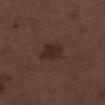Captured during whole-body skin photography for melanoma surveillance; the lesion was not biopsied. Captured under white-light illumination. About 2.5 mm across. The subject is a male aged approximately 70. A 15 mm close-up extracted from a 3D total-body photography capture. The lesion is located on the right lower leg.